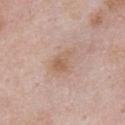Impression:
Imaged during a routine full-body skin examination; the lesion was not biopsied and no histopathology is available.
Image and clinical context:
Approximately 3 mm at its widest. The lesion is located on the front of the torso. Automated image analysis of the tile measured a symmetry-axis asymmetry near 0.3. And it measured an average lesion color of about L≈60 a*≈18 b*≈29 (CIELAB) and about 7 CIELAB-L* units darker than the surrounding skin. The software also gave a border-irregularity index near 3/10, internal color variation of about 3.5 on a 0–10 scale, and radial color variation of about 1.5. The analysis additionally found a lesion-detection confidence of about 100/100. The patient is a male about 55 years old. A 15 mm close-up tile from a total-body photography series done for melanoma screening.